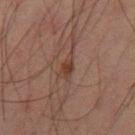The lesion was tiled from a total-body skin photograph and was not biopsied. Cropped from a whole-body photographic skin survey; the tile spans about 15 mm. The lesion is on the left thigh. A male subject, aged approximately 60. Imaged with cross-polarized lighting. The recorded lesion diameter is about 3.5 mm. The total-body-photography lesion software estimated an area of roughly 4 mm² and a symmetry-axis asymmetry near 0.3. The software also gave a normalized lesion–skin contrast near 7. And it measured a nevus-likeness score of about 35/100.